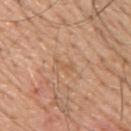Impression:
No biopsy was performed on this lesion — it was imaged during a full skin examination and was not determined to be concerning.
Context:
Automated tile analysis of the lesion measured a color-variation rating of about 0/10 and peripheral color asymmetry of about 0. And it measured a nevus-likeness score of about 0/100 and a lesion-detection confidence of about 85/100. About 2.5 mm across. On the back. Imaged with white-light lighting. A roughly 15 mm field-of-view crop from a total-body skin photograph. A male subject, aged 53 to 57.